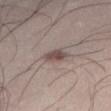The lesion was photographed on a routine skin check and not biopsied; there is no pathology result.
Cropped from a total-body skin-imaging series; the visible field is about 15 mm.
The lesion is on the right thigh.
The total-body-photography lesion software estimated border irregularity of about 2 on a 0–10 scale, internal color variation of about 2.5 on a 0–10 scale, and radial color variation of about 1. The analysis additionally found a lesion-detection confidence of about 100/100.
The lesion's longest dimension is about 3 mm.
The subject is a male aged 23 to 27.
Captured under white-light illumination.